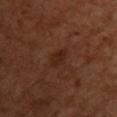biopsy status: total-body-photography surveillance lesion; no biopsy | image source: ~15 mm crop, total-body skin-cancer survey | subject: female, in their mid- to late 50s | size: about 3 mm | anatomic site: the upper back.